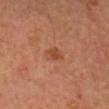• biopsy status: imaged on a skin check; not biopsied
• image: ~15 mm crop, total-body skin-cancer survey
• subject: female, aged 38 to 42
• body site: the head or neck
• illumination: cross-polarized
• automated metrics: an eccentricity of roughly 0.65